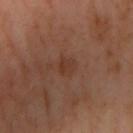biopsy_status: not biopsied; imaged during a skin examination
image:
  source: total-body photography crop
  field_of_view_mm: 15
lesion_size:
  long_diameter_mm_approx: 2.5
patient:
  sex: female
  age_approx: 55
site: right upper arm